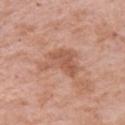No biopsy was performed on this lesion — it was imaged during a full skin examination and was not determined to be concerning. A female patient aged 68 to 72. A 15 mm crop from a total-body photograph taken for skin-cancer surveillance. Imaged with white-light lighting. The lesion is located on the left upper arm.